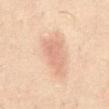Imaged during a routine full-body skin examination; the lesion was not biopsied and no histopathology is available.
About 4 mm across.
This is a cross-polarized tile.
A male patient, aged 48–52.
Located on the abdomen.
A close-up tile cropped from a whole-body skin photograph, about 15 mm across.
Automated image analysis of the tile measured an area of roughly 8 mm², a shape eccentricity near 0.85, and two-axis asymmetry of about 0.3. The software also gave about 8 CIELAB-L* units darker than the surrounding skin and a normalized lesion–skin contrast near 5. And it measured an automated nevus-likeness rating near 55 out of 100 and a detector confidence of about 100 out of 100 that the crop contains a lesion.